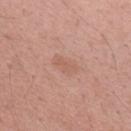This lesion was catalogued during total-body skin photography and was not selected for biopsy. Cropped from a total-body skin-imaging series; the visible field is about 15 mm. On the right upper arm. A female patient, in their mid- to late 30s. Imaged with white-light lighting.